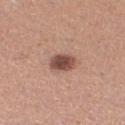<tbp_lesion>
<biopsy_status>not biopsied; imaged during a skin examination</biopsy_status>
<lighting>white-light</lighting>
<site>right thigh</site>
<patient>
  <sex>female</sex>
  <age_approx>30</age_approx>
</patient>
<image>
  <source>total-body photography crop</source>
  <field_of_view_mm>15</field_of_view_mm>
</image>
</tbp_lesion>